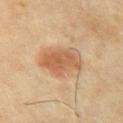Clinical impression:
The lesion was photographed on a routine skin check and not biopsied; there is no pathology result.
Background:
Cropped from a whole-body photographic skin survey; the tile spans about 15 mm. A male subject about 65 years old. From the arm.